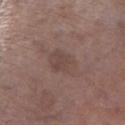Impression:
No biopsy was performed on this lesion — it was imaged during a full skin examination and was not determined to be concerning.
Context:
Located on the left thigh. A male patient, in their mid-70s. Automated image analysis of the tile measured a lesion area of about 8 mm², an outline eccentricity of about 0.55 (0 = round, 1 = elongated), and two-axis asymmetry of about 0.15. It also reported a classifier nevus-likeness of about 0/100 and lesion-presence confidence of about 100/100. Cropped from a whole-body photographic skin survey; the tile spans about 15 mm.